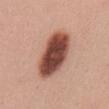No biopsy was performed on this lesion — it was imaged during a full skin examination and was not determined to be concerning.
This image is a 15 mm lesion crop taken from a total-body photograph.
The lesion is located on the abdomen.
The patient is a female about 40 years old.